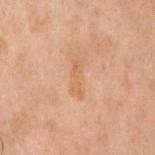Recorded during total-body skin imaging; not selected for excision or biopsy.
On the chest.
The recorded lesion diameter is about 4 mm.
This is a cross-polarized tile.
A 15 mm close-up tile from a total-body photography series done for melanoma screening.
The subject is a male aged around 70.
The lesion-visualizer software estimated a shape eccentricity near 0.95 and a shape-asymmetry score of about 0.3 (0 = symmetric).